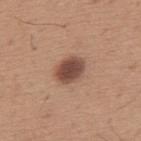The total-body-photography lesion software estimated a footprint of about 8 mm² and a shape eccentricity near 0.65. And it measured a border-irregularity index near 1.5/10 and a peripheral color-asymmetry measure near 1. It also reported an automated nevus-likeness rating near 80 out of 100 and a lesion-detection confidence of about 100/100. A 15 mm crop from a total-body photograph taken for skin-cancer surveillance. A male subject, aged approximately 65. Captured under white-light illumination. The lesion is located on the upper back.